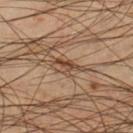Notes:
• biopsy status · imaged on a skin check; not biopsied
• anatomic site · the left lower leg
• acquisition · ~15 mm crop, total-body skin-cancer survey
• lesion diameter · ~3.5 mm (longest diameter)
• lighting · cross-polarized illumination
• TBP lesion metrics · an outline eccentricity of about 0.9 (0 = round, 1 = elongated) and a shape-asymmetry score of about 0.4 (0 = symmetric); a lesion color around L≈45 a*≈17 b*≈29 in CIELAB, about 9 CIELAB-L* units darker than the surrounding skin, and a normalized border contrast of about 7.5; border irregularity of about 4.5 on a 0–10 scale, a within-lesion color-variation index near 6.5/10, and radial color variation of about 2.5; a classifier nevus-likeness of about 0/100 and a lesion-detection confidence of about 0/100
• subject · male, about 45 years old